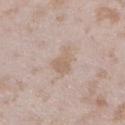notes: no biopsy performed (imaged during a skin exam); imaging modality: 15 mm crop, total-body photography; patient: female, aged 23–27; diameter: ~3 mm (longest diameter); lighting: white-light; body site: the left lower leg.